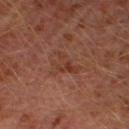| feature | finding |
|---|---|
| biopsy status | imaged on a skin check; not biopsied |
| image | 15 mm crop, total-body photography |
| subject | male, about 30 years old |
| lesion diameter | ~3.5 mm (longest diameter) |
| illumination | cross-polarized illumination |
| automated metrics | a lesion area of about 6 mm², a shape eccentricity near 0.75, and a shape-asymmetry score of about 0.5 (0 = symmetric); an average lesion color of about L≈37 a*≈23 b*≈28 (CIELAB), about 5 CIELAB-L* units darker than the surrounding skin, and a normalized border contrast of about 5.5; an automated nevus-likeness rating near 0 out of 100 and a detector confidence of about 100 out of 100 that the crop contains a lesion |
| site | the left leg |